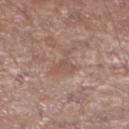Clinical impression: Captured during whole-body skin photography for melanoma surveillance; the lesion was not biopsied. Clinical summary: Cropped from a whole-body photographic skin survey; the tile spans about 15 mm. The lesion is on the left lower leg. A male patient, in their mid- to late 60s.